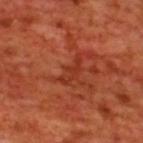Notes:
- follow-up — catalogued during a skin exam; not biopsied
- site — the upper back
- tile lighting — cross-polarized
- imaging modality — 15 mm crop, total-body photography
- lesion diameter — ~3.5 mm (longest diameter)
- subject — male, aged 68 to 72